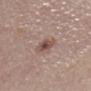Case summary:
– image source · 15 mm crop, total-body photography
– diameter · ~2.5 mm (longest diameter)
– location · the mid back
– automated metrics · an area of roughly 5.5 mm², an outline eccentricity of about 0.3 (0 = round, 1 = elongated), and two-axis asymmetry of about 0.2; a border-irregularity rating of about 2.5/10 and radial color variation of about 1.5
– subject · male, in their mid- to late 50s